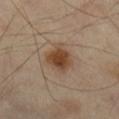Assessment:
No biopsy was performed on this lesion — it was imaged during a full skin examination and was not determined to be concerning.
Image and clinical context:
The lesion is on the right lower leg. This image is a 15 mm lesion crop taken from a total-body photograph. A male subject aged 53 to 57.